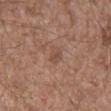Clinical impression:
This lesion was catalogued during total-body skin photography and was not selected for biopsy.
Clinical summary:
A male patient aged 73 to 77. Imaged with white-light lighting. This image is a 15 mm lesion crop taken from a total-body photograph. From the mid back.